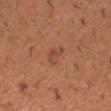notes: total-body-photography surveillance lesion; no biopsy | location: the arm | size: about 2.5 mm | automated metrics: border irregularity of about 3.5 on a 0–10 scale, a within-lesion color-variation index near 1.5/10, and a peripheral color-asymmetry measure near 0.5; a classifier nevus-likeness of about 35/100 | patient: male, in their 40s | imaging modality: ~15 mm crop, total-body skin-cancer survey.